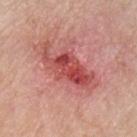This lesion was catalogued during total-body skin photography and was not selected for biopsy. A roughly 15 mm field-of-view crop from a total-body skin photograph. The subject is a male roughly 80 years of age. The lesion is on the chest.